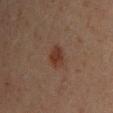Impression: Part of a total-body skin-imaging series; this lesion was reviewed on a skin check and was not flagged for biopsy. Image and clinical context: Cropped from a whole-body photographic skin survey; the tile spans about 15 mm. A male patient roughly 30 years of age. Automated tile analysis of the lesion measured an eccentricity of roughly 0.55. The software also gave a nevus-likeness score of about 90/100 and a detector confidence of about 100 out of 100 that the crop contains a lesion. The recorded lesion diameter is about 2.5 mm. From the front of the torso.